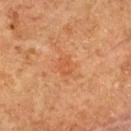Impression:
Recorded during total-body skin imaging; not selected for excision or biopsy.
Context:
Automated tile analysis of the lesion measured border irregularity of about 2.5 on a 0–10 scale and internal color variation of about 1.5 on a 0–10 scale. And it measured an automated nevus-likeness rating near 0 out of 100 and a lesion-detection confidence of about 100/100. A female patient, aged 58 to 62. Captured under cross-polarized illumination. From the upper back. Cropped from a whole-body photographic skin survey; the tile spans about 15 mm.